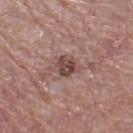Clinical impression: The lesion was tiled from a total-body skin photograph and was not biopsied. Acquisition and patient details: Approximately 3 mm at its widest. Located on the right thigh. This is a white-light tile. A female patient, aged approximately 70. A 15 mm crop from a total-body photograph taken for skin-cancer surveillance.